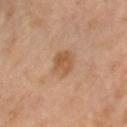This lesion was catalogued during total-body skin photography and was not selected for biopsy. Cropped from a whole-body photographic skin survey; the tile spans about 15 mm. A female patient, in their 60s. The lesion's longest dimension is about 3.5 mm. The lesion is located on the chest. The tile uses cross-polarized illumination.